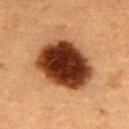Notes:
• automated metrics — a footprint of about 30 mm², a shape eccentricity near 0.65, and a symmetry-axis asymmetry near 0.1; an average lesion color of about L≈29 a*≈22 b*≈28 (CIELAB) and a normalized lesion–skin contrast near 19; an automated nevus-likeness rating near 95 out of 100 and lesion-presence confidence of about 100/100
• subject — female, roughly 55 years of age
• body site — the upper back
• lighting — cross-polarized illumination
• size — about 7 mm
• image source — ~15 mm tile from a whole-body skin photo